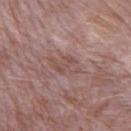notes = catalogued during a skin exam; not biopsied
acquisition = total-body-photography crop, ~15 mm field of view
tile lighting = white-light illumination
anatomic site = the right forearm
patient = male, aged around 40
diameter = ≈4 mm